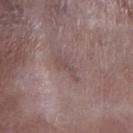Clinical impression:
Recorded during total-body skin imaging; not selected for excision or biopsy.
Background:
The subject is a male roughly 75 years of age. A roughly 15 mm field-of-view crop from a total-body skin photograph. On the right lower leg.